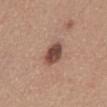The lesion was photographed on a routine skin check and not biopsied; there is no pathology result. Imaged with white-light lighting. Located on the mid back. A male patient, approximately 55 years of age. An algorithmic analysis of the crop reported border irregularity of about 2 on a 0–10 scale, a within-lesion color-variation index near 5/10, and a peripheral color-asymmetry measure near 1.5. It also reported an automated nevus-likeness rating near 75 out of 100. The lesion's longest dimension is about 3 mm. Cropped from a whole-body photographic skin survey; the tile spans about 15 mm.